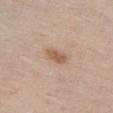Imaged during a routine full-body skin examination; the lesion was not biopsied and no histopathology is available. Cropped from a whole-body photographic skin survey; the tile spans about 15 mm. The subject is a female aged around 65. Located on the chest.